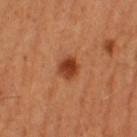Imaged during a routine full-body skin examination; the lesion was not biopsied and no histopathology is available. The patient is a female approximately 55 years of age. The recorded lesion diameter is about 3 mm. Automated image analysis of the tile measured an area of roughly 6 mm², a shape eccentricity near 0.25, and a shape-asymmetry score of about 0.2 (0 = symmetric). The software also gave a lesion color around L≈43 a*≈29 b*≈38 in CIELAB, roughly 13 lightness units darker than nearby skin, and a normalized lesion–skin contrast near 9.5. The software also gave border irregularity of about 1.5 on a 0–10 scale and a peripheral color-asymmetry measure near 1.5. This image is a 15 mm lesion crop taken from a total-body photograph. On the leg.